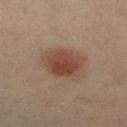Recorded during total-body skin imaging; not selected for excision or biopsy. The tile uses cross-polarized illumination. The lesion is on the left leg. A female subject aged 33 to 37. Cropped from a total-body skin-imaging series; the visible field is about 15 mm.